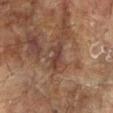Case summary:
• workup · imaged on a skin check; not biopsied
• diameter · about 3.5 mm
• tile lighting · cross-polarized
• image-analysis metrics · a footprint of about 3.5 mm², an eccentricity of roughly 0.95, and a symmetry-axis asymmetry near 0.3; a mean CIELAB color near L≈35 a*≈18 b*≈23 and roughly 7 lightness units darker than nearby skin
• subject · female, approximately 80 years of age
• image · 15 mm crop, total-body photography
• anatomic site · the left arm